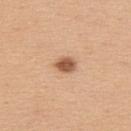body site: the upper back; image source: ~15 mm tile from a whole-body skin photo; patient: male, about 35 years old; automated lesion analysis: an automated nevus-likeness rating near 95 out of 100; lighting: white-light.